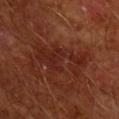follow-up: total-body-photography surveillance lesion; no biopsy | acquisition: 15 mm crop, total-body photography | lesion diameter: about 6.5 mm | subject: male, approximately 65 years of age | TBP lesion metrics: a mean CIELAB color near L≈25 a*≈24 b*≈26, about 5 CIELAB-L* units darker than the surrounding skin, and a lesion-to-skin contrast of about 6 (normalized; higher = more distinct); a border-irregularity index near 8/10 | tile lighting: cross-polarized illumination | body site: the right upper arm.